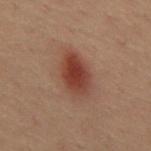Q: Is there a histopathology result?
A: imaged on a skin check; not biopsied
Q: How was this image acquired?
A: ~15 mm crop, total-body skin-cancer survey
Q: Lesion size?
A: ≈5 mm
Q: Who is the patient?
A: male, aged approximately 40
Q: How was the tile lit?
A: cross-polarized illumination
Q: Lesion location?
A: the mid back
Q: Automated lesion metrics?
A: a lesion area of about 11 mm², a shape eccentricity near 0.85, and a shape-asymmetry score of about 0.15 (0 = symmetric); a mean CIELAB color near L≈32 a*≈20 b*≈23, a lesion–skin lightness drop of about 10, and a normalized border contrast of about 9.5; a classifier nevus-likeness of about 100/100 and a detector confidence of about 100 out of 100 that the crop contains a lesion